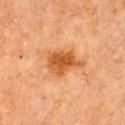This lesion was catalogued during total-body skin photography and was not selected for biopsy. Imaged with cross-polarized lighting. A 15 mm close-up extracted from a 3D total-body photography capture. From the chest. The lesion-visualizer software estimated an eccentricity of roughly 0.75 and a shape-asymmetry score of about 0.35 (0 = symmetric). It also reported a mean CIELAB color near L≈50 a*≈26 b*≈41. It also reported a border-irregularity index near 3.5/10, a color-variation rating of about 4/10, and radial color variation of about 1.5. It also reported an automated nevus-likeness rating near 80 out of 100 and a detector confidence of about 100 out of 100 that the crop contains a lesion. A female subject aged 58–62. The recorded lesion diameter is about 5 mm.